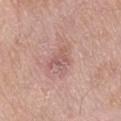The lesion was tiled from a total-body skin photograph and was not biopsied.
From the leg.
Cropped from a total-body skin-imaging series; the visible field is about 15 mm.
A male patient approximately 80 years of age.
Imaged with white-light lighting.
Longest diameter approximately 3.5 mm.